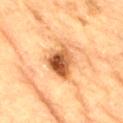The lesion was tiled from a total-body skin photograph and was not biopsied. About 5 mm across. A male subject approximately 85 years of age or older. Automated tile analysis of the lesion measured a mean CIELAB color near L≈53 a*≈24 b*≈39 and a normalized border contrast of about 10.5. It also reported a border-irregularity index near 3.5/10, internal color variation of about 10 on a 0–10 scale, and peripheral color asymmetry of about 3.5. It also reported an automated nevus-likeness rating near 70 out of 100 and a lesion-detection confidence of about 100/100. On the back. A close-up tile cropped from a whole-body skin photograph, about 15 mm across. This is a cross-polarized tile.